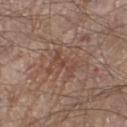This lesion was catalogued during total-body skin photography and was not selected for biopsy. The lesion's longest dimension is about 3.5 mm. A lesion tile, about 15 mm wide, cut from a 3D total-body photograph. The tile uses white-light illumination. The lesion is located on the left thigh. Automated image analysis of the tile measured about 6 CIELAB-L* units darker than the surrounding skin and a normalized lesion–skin contrast near 5. It also reported a classifier nevus-likeness of about 0/100 and a detector confidence of about 60 out of 100 that the crop contains a lesion. The patient is a male about 80 years old.